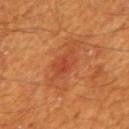Part of a total-body skin-imaging series; this lesion was reviewed on a skin check and was not flagged for biopsy.
On the mid back.
Cropped from a whole-body photographic skin survey; the tile spans about 15 mm.
A male subject aged 58 to 62.
The total-body-photography lesion software estimated a lesion color around L≈43 a*≈31 b*≈36 in CIELAB, a lesion–skin lightness drop of about 6, and a normalized lesion–skin contrast near 5.5.